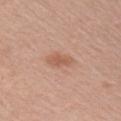| key | value |
|---|---|
| notes | total-body-photography surveillance lesion; no biopsy |
| tile lighting | white-light |
| TBP lesion metrics | a border-irregularity rating of about 2.5/10 and a color-variation rating of about 2/10 |
| diameter | ~3 mm (longest diameter) |
| location | the right upper arm |
| image source | 15 mm crop, total-body photography |
| patient | male, aged approximately 60 |